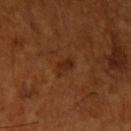notes = catalogued during a skin exam; not biopsied
acquisition = 15 mm crop, total-body photography
location = the left upper arm
illumination = cross-polarized illumination
diameter = about 2.5 mm
subject = male, aged 63–67